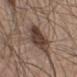Recorded during total-body skin imaging; not selected for excision or biopsy. Cropped from a total-body skin-imaging series; the visible field is about 15 mm. About 7.5 mm across. A male patient, in their 60s. The tile uses white-light illumination. On the right lower leg.